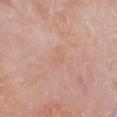Case summary:
- biopsy status · total-body-photography surveillance lesion; no biopsy
- automated lesion analysis · a lesion area of about 3.5 mm²; a lesion color around L≈64 a*≈20 b*≈29 in CIELAB, a lesion–skin lightness drop of about 4, and a lesion-to-skin contrast of about 3 (normalized; higher = more distinct); a border-irregularity rating of about 3.5/10 and a peripheral color-asymmetry measure near 0.5
- lesion size · about 3 mm
- imaging modality · 15 mm crop, total-body photography
- lighting · white-light
- patient · female, roughly 50 years of age
- anatomic site · the leg